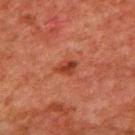Recorded during total-body skin imaging; not selected for excision or biopsy. A 15 mm close-up tile from a total-body photography series done for melanoma screening. A male subject, aged approximately 80. The lesion is located on the mid back.